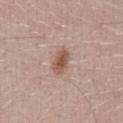Captured under white-light illumination. The lesion's longest dimension is about 3.5 mm. A 15 mm close-up extracted from a 3D total-body photography capture. Automated image analysis of the tile measured a footprint of about 5 mm² and two-axis asymmetry of about 0.25. A male subject, aged 28–32.